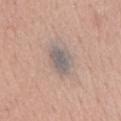Q: Was this lesion biopsied?
A: imaged on a skin check; not biopsied
Q: What are the patient's age and sex?
A: male, in their mid- to late 50s
Q: How was this image acquired?
A: ~15 mm tile from a whole-body skin photo